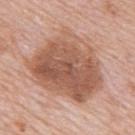This lesion was catalogued during total-body skin photography and was not selected for biopsy. The tile uses white-light illumination. Located on the upper back. Longest diameter approximately 9 mm. A roughly 15 mm field-of-view crop from a total-body skin photograph. The subject is a male about 80 years old.